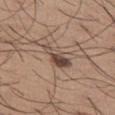Captured during whole-body skin photography for melanoma surveillance; the lesion was not biopsied. The lesion is on the left thigh. The subject is a male aged around 65. A 15 mm close-up tile from a total-body photography series done for melanoma screening.